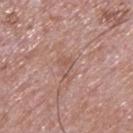Part of a total-body skin-imaging series; this lesion was reviewed on a skin check and was not flagged for biopsy. A male subject aged approximately 65. The lesion is on the upper back. A close-up tile cropped from a whole-body skin photograph, about 15 mm across. Imaged with white-light lighting. Longest diameter approximately 3 mm.